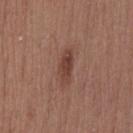Clinical impression:
The lesion was photographed on a routine skin check and not biopsied; there is no pathology result.
Background:
On the lower back. The recorded lesion diameter is about 4 mm. The tile uses white-light illumination. Cropped from a whole-body photographic skin survey; the tile spans about 15 mm. A female patient, aged 38–42.